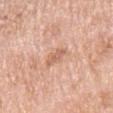Impression: Captured during whole-body skin photography for melanoma surveillance; the lesion was not biopsied. Background: Captured under white-light illumination. A male subject roughly 60 years of age. A region of skin cropped from a whole-body photographic capture, roughly 15 mm wide. The recorded lesion diameter is about 2.5 mm. The lesion is located on the right upper arm. An algorithmic analysis of the crop reported an average lesion color of about L≈63 a*≈22 b*≈32 (CIELAB), a lesion–skin lightness drop of about 8, and a normalized lesion–skin contrast near 5.5. The software also gave border irregularity of about 3.5 on a 0–10 scale, a color-variation rating of about 1.5/10, and a peripheral color-asymmetry measure near 0.5.